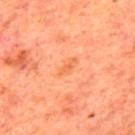Notes:
- follow-up: catalogued during a skin exam; not biopsied
- site: the mid back
- lesion diameter: about 3 mm
- imaging modality: ~15 mm crop, total-body skin-cancer survey
- illumination: cross-polarized
- subject: male, in their mid- to late 60s
- automated metrics: an area of roughly 3 mm² and a shape eccentricity near 0.9; a mean CIELAB color near L≈60 a*≈30 b*≈42; a peripheral color-asymmetry measure near 0.5